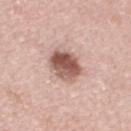Clinical impression: No biopsy was performed on this lesion — it was imaged during a full skin examination and was not determined to be concerning. Acquisition and patient details: Captured under white-light illumination. A 15 mm crop from a total-body photograph taken for skin-cancer surveillance. Measured at roughly 4 mm in maximum diameter. Located on the left upper arm. The total-body-photography lesion software estimated roughly 17 lightness units darker than nearby skin and a normalized lesion–skin contrast near 10.5. The software also gave a within-lesion color-variation index near 7/10 and radial color variation of about 2.5. The analysis additionally found lesion-presence confidence of about 100/100. A female patient aged approximately 50.